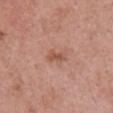Part of a total-body skin-imaging series; this lesion was reviewed on a skin check and was not flagged for biopsy.
A female subject aged around 45.
The lesion is located on the chest.
A region of skin cropped from a whole-body photographic capture, roughly 15 mm wide.
This is a white-light tile.